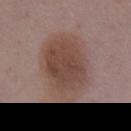| field | value |
|---|---|
| notes | no biopsy performed (imaged during a skin exam) |
| automated lesion analysis | a lesion color around L≈44 a*≈18 b*≈24 in CIELAB and a normalized lesion–skin contrast near 7.5 |
| subject | female, aged approximately 50 |
| tile lighting | white-light |
| anatomic site | the chest |
| size | ~6 mm (longest diameter) |
| acquisition | ~15 mm tile from a whole-body skin photo |